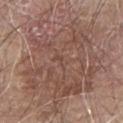notes = total-body-photography surveillance lesion; no biopsy | subject = male, aged 78 to 82 | acquisition = ~15 mm crop, total-body skin-cancer survey | tile lighting = white-light | location = the left arm | lesion size = about 12.5 mm.